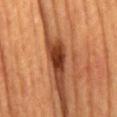Imaged during a routine full-body skin examination; the lesion was not biopsied and no histopathology is available. This is a cross-polarized tile. A male subject in their mid-60s. Longest diameter approximately 3 mm. Cropped from a whole-body photographic skin survey; the tile spans about 15 mm. The lesion is on the front of the torso. Automated image analysis of the tile measured a footprint of about 5 mm², an outline eccentricity of about 0.85 (0 = round, 1 = elongated), and a symmetry-axis asymmetry near 0.2. It also reported a lesion color around L≈33 a*≈27 b*≈33 in CIELAB, a lesion–skin lightness drop of about 18, and a normalized lesion–skin contrast near 14. And it measured a nevus-likeness score of about 100/100 and a lesion-detection confidence of about 100/100.